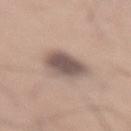Captured under white-light illumination. An algorithmic analysis of the crop reported a border-irregularity rating of about 2/10, internal color variation of about 4.5 on a 0–10 scale, and radial color variation of about 1. About 4.5 mm across. This image is a 15 mm lesion crop taken from a total-body photograph. Located on the lower back. The subject is a male in their mid-60s.